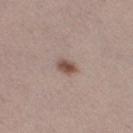Clinical impression:
The lesion was tiled from a total-body skin photograph and was not biopsied.
Context:
Measured at roughly 2.5 mm in maximum diameter. The tile uses white-light illumination. The lesion-visualizer software estimated an area of roughly 4 mm² and a shape-asymmetry score of about 0.25 (0 = symmetric). The software also gave a lesion color around L≈51 a*≈17 b*≈24 in CIELAB, about 12 CIELAB-L* units darker than the surrounding skin, and a normalized lesion–skin contrast near 9. The analysis additionally found a border-irregularity rating of about 2/10 and a peripheral color-asymmetry measure near 1. It also reported a classifier nevus-likeness of about 95/100. A female subject aged 38–42. A region of skin cropped from a whole-body photographic capture, roughly 15 mm wide. The lesion is on the left lower leg.